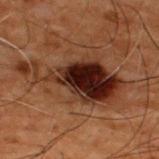| feature | finding |
|---|---|
| workup | catalogued during a skin exam; not biopsied |
| location | the upper back |
| patient | male, aged approximately 50 |
| imaging modality | ~15 mm tile from a whole-body skin photo |